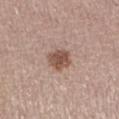Assessment: Recorded during total-body skin imaging; not selected for excision or biopsy. Image and clinical context: A female subject, aged approximately 35. Cropped from a total-body skin-imaging series; the visible field is about 15 mm. The lesion-visualizer software estimated a mean CIELAB color near L≈51 a*≈19 b*≈26 and roughly 13 lightness units darker than nearby skin. The recorded lesion diameter is about 3 mm. Located on the left lower leg.